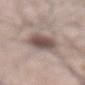Background: The subject is a male roughly 65 years of age. This is a white-light tile. The lesion is located on the abdomen. Measured at roughly 6.5 mm in maximum diameter. A 15 mm close-up tile from a total-body photography series done for melanoma screening. An algorithmic analysis of the crop reported an area of roughly 14 mm², an outline eccentricity of about 0.9 (0 = round, 1 = elongated), and a symmetry-axis asymmetry near 0.2. It also reported a lesion color around L≈52 a*≈13 b*≈20 in CIELAB, a lesion–skin lightness drop of about 14, and a normalized border contrast of about 9.5. The analysis additionally found border irregularity of about 3.5 on a 0–10 scale, a within-lesion color-variation index near 5.5/10, and radial color variation of about 1.5. And it measured a classifier nevus-likeness of about 95/100.